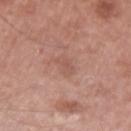Q: Was this lesion biopsied?
A: total-body-photography surveillance lesion; no biopsy
Q: Illumination type?
A: white-light illumination
Q: How was this image acquired?
A: ~15 mm crop, total-body skin-cancer survey
Q: Who is the patient?
A: male, aged approximately 65
Q: Where on the body is the lesion?
A: the arm